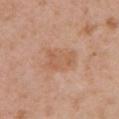Background: On the front of the torso. This is a white-light tile. A female patient, aged approximately 40. A lesion tile, about 15 mm wide, cut from a 3D total-body photograph. The lesion-visualizer software estimated border irregularity of about 3 on a 0–10 scale, a color-variation rating of about 2.5/10, and peripheral color asymmetry of about 0.5. It also reported a nevus-likeness score of about 0/100 and a detector confidence of about 100 out of 100 that the crop contains a lesion. Approximately 4 mm at its widest.